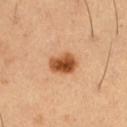Recorded during total-body skin imaging; not selected for excision or biopsy. Automated tile analysis of the lesion measured a lesion color around L≈51 a*≈26 b*≈40 in CIELAB, roughly 17 lightness units darker than nearby skin, and a normalized border contrast of about 12. The analysis additionally found a within-lesion color-variation index near 6/10. The tile uses cross-polarized illumination. A male subject roughly 50 years of age. This image is a 15 mm lesion crop taken from a total-body photograph. Longest diameter approximately 3.5 mm. From the right thigh.